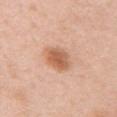Notes:
- patient — female, aged 48 to 52
- automated lesion analysis — an outline eccentricity of about 0.6 (0 = round, 1 = elongated) and a symmetry-axis asymmetry near 0.15; a border-irregularity index near 1.5/10, internal color variation of about 4 on a 0–10 scale, and radial color variation of about 1.5
- site — the left upper arm
- size — about 3.5 mm
- illumination — white-light illumination
- acquisition — ~15 mm crop, total-body skin-cancer survey